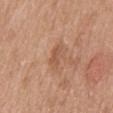biopsy_status: not biopsied; imaged during a skin examination
image:
  source: total-body photography crop
  field_of_view_mm: 15
automated_metrics:
  cielab_L: 54
  cielab_a: 22
  cielab_b: 33
  vs_skin_darker_L: 8.0
  border_irregularity_0_10: 4.0
  color_variation_0_10: 1.5
  peripheral_color_asymmetry: 0.5
patient:
  sex: female
  age_approx: 40
site: mid back